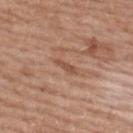{
  "biopsy_status": "not biopsied; imaged during a skin examination",
  "lighting": "white-light",
  "image": {
    "source": "total-body photography crop",
    "field_of_view_mm": 15
  },
  "lesion_size": {
    "long_diameter_mm_approx": 2.5
  },
  "patient": {
    "sex": "female",
    "age_approx": 60
  },
  "site": "upper back"
}